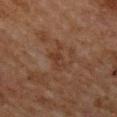The patient is a female aged around 60. The recorded lesion diameter is about 4 mm. This is a cross-polarized tile. A close-up tile cropped from a whole-body skin photograph, about 15 mm across. Automated image analysis of the tile measured border irregularity of about 6 on a 0–10 scale and a within-lesion color-variation index near 2.5/10. It also reported a classifier nevus-likeness of about 0/100 and a detector confidence of about 95 out of 100 that the crop contains a lesion. Located on the upper back.